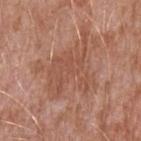The patient is a male aged around 45. The lesion is on the left upper arm. The tile uses white-light illumination. A 15 mm close-up extracted from a 3D total-body photography capture.